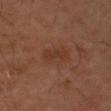Image and clinical context:
Cropped from a whole-body photographic skin survey; the tile spans about 15 mm. Imaged with cross-polarized lighting. A male patient roughly 50 years of age. From the left arm.